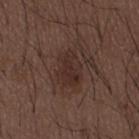No biopsy was performed on this lesion — it was imaged during a full skin examination and was not determined to be concerning.
The lesion is on the mid back.
A close-up tile cropped from a whole-body skin photograph, about 15 mm across.
Captured under white-light illumination.
A male subject, roughly 50 years of age.